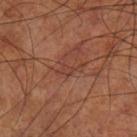imaging modality = total-body-photography crop, ~15 mm field of view; subject = male, aged around 70; body site = the left lower leg.